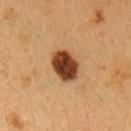Recorded during total-body skin imaging; not selected for excision or biopsy. Located on the left upper arm. Automated image analysis of the tile measured a footprint of about 9.5 mm², an eccentricity of roughly 0.7, and a shape-asymmetry score of about 0.15 (0 = symmetric). This image is a 15 mm lesion crop taken from a total-body photograph. The subject is a female in their 40s.